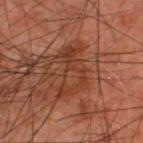Q: Is there a histopathology result?
A: imaged on a skin check; not biopsied
Q: Automated lesion metrics?
A: a border-irregularity rating of about 8.5/10, a color-variation rating of about 4.5/10, and a peripheral color-asymmetry measure near 1; an automated nevus-likeness rating near 0 out of 100 and lesion-presence confidence of about 100/100
Q: How was this image acquired?
A: 15 mm crop, total-body photography
Q: Patient demographics?
A: male, roughly 60 years of age
Q: Lesion location?
A: the upper back
Q: What lighting was used for the tile?
A: cross-polarized